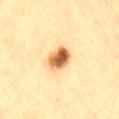No biopsy was performed on this lesion — it was imaged during a full skin examination and was not determined to be concerning. The tile uses cross-polarized illumination. A roughly 15 mm field-of-view crop from a total-body skin photograph. The subject is a male about 85 years old or older. Located on the lower back. The recorded lesion diameter is about 3.5 mm. Automated image analysis of the tile measured a footprint of about 7.5 mm², a shape eccentricity near 0.5, and a shape-asymmetry score of about 0.15 (0 = symmetric). It also reported a nevus-likeness score of about 100/100 and a detector confidence of about 100 out of 100 that the crop contains a lesion.